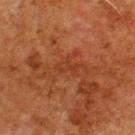site: upper back
patient:
  sex: male
  age_approx: 80
image:
  source: total-body photography crop
  field_of_view_mm: 15
lesion_size:
  long_diameter_mm_approx: 2.5
automated_metrics:
  area_mm2_approx: 3.0
  eccentricity: 0.9
  shape_asymmetry: 0.4